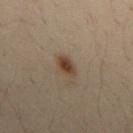{"biopsy_status": "not biopsied; imaged during a skin examination", "site": "mid back", "automated_metrics": {"cielab_L": 32, "cielab_a": 14, "cielab_b": 23, "vs_skin_darker_L": 9.0, "vs_skin_contrast_norm": 9.5, "border_irregularity_0_10": 3.0, "color_variation_0_10": 2.5, "peripheral_color_asymmetry": 0.5}, "lighting": "cross-polarized", "patient": {"sex": "male", "age_approx": 30}, "image": {"source": "total-body photography crop", "field_of_view_mm": 15}}